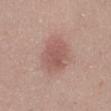Clinical impression: The lesion was photographed on a routine skin check and not biopsied; there is no pathology result. Image and clinical context: A close-up tile cropped from a whole-body skin photograph, about 15 mm across. Automated tile analysis of the lesion measured internal color variation of about 2.5 on a 0–10 scale and a peripheral color-asymmetry measure near 0.5. It also reported a classifier nevus-likeness of about 55/100 and a lesion-detection confidence of about 100/100. Approximately 4 mm at its widest. A female subject in their 20s. The lesion is on the leg.